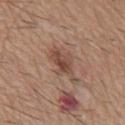Case summary:
• notes · total-body-photography surveillance lesion; no biopsy
• image source · ~15 mm tile from a whole-body skin photo
• TBP lesion metrics · a footprint of about 8 mm², an outline eccentricity of about 0.9 (0 = round, 1 = elongated), and a shape-asymmetry score of about 0.3 (0 = symmetric); border irregularity of about 4.5 on a 0–10 scale and radial color variation of about 1.5; a classifier nevus-likeness of about 40/100 and a detector confidence of about 100 out of 100 that the crop contains a lesion
• diameter · about 5 mm
• body site · the mid back
• tile lighting · white-light illumination
• subject · male, aged around 60